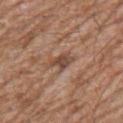No biopsy was performed on this lesion — it was imaged during a full skin examination and was not determined to be concerning.
The lesion-visualizer software estimated a footprint of about 5 mm². It also reported a mean CIELAB color near L≈47 a*≈19 b*≈27, roughly 10 lightness units darker than nearby skin, and a lesion-to-skin contrast of about 7.5 (normalized; higher = more distinct). And it measured a border-irregularity rating of about 3.5/10 and peripheral color asymmetry of about 0.5.
On the right upper arm.
A 15 mm crop from a total-body photograph taken for skin-cancer surveillance.
The tile uses white-light illumination.
A male patient aged 58–62.